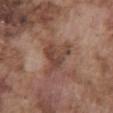Clinical impression:
The lesion was tiled from a total-body skin photograph and was not biopsied.
Image and clinical context:
A 15 mm crop from a total-body photograph taken for skin-cancer surveillance. Measured at roughly 5 mm in maximum diameter. A male subject aged around 75. Located on the abdomen. This is a white-light tile.